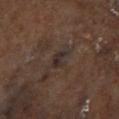Notes:
– notes · total-body-photography surveillance lesion; no biopsy
– image source · ~15 mm tile from a whole-body skin photo
– lighting · cross-polarized
– subject · male, aged 68 to 72
– anatomic site · the right lower leg
– automated lesion analysis · a lesion area of about 3 mm² and a shape eccentricity near 0.75; an average lesion color of about L≈25 a*≈10 b*≈16 (CIELAB) and about 6 CIELAB-L* units darker than the surrounding skin; a within-lesion color-variation index near 1/10 and peripheral color asymmetry of about 0
– size · ≈2.5 mm